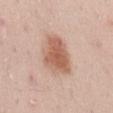{
  "image": {
    "source": "total-body photography crop",
    "field_of_view_mm": 15
  },
  "lighting": "white-light",
  "automated_metrics": {
    "border_irregularity_0_10": 2.5
  },
  "site": "lower back",
  "patient": {
    "sex": "male",
    "age_approx": 50
  }
}